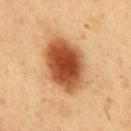follow-up — imaged on a skin check; not biopsied | diameter — ~7 mm (longest diameter) | acquisition — total-body-photography crop, ~15 mm field of view | location — the abdomen | tile lighting — cross-polarized illumination | subject — male, approximately 50 years of age.